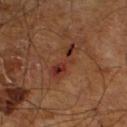notes: total-body-photography surveillance lesion; no biopsy
automated metrics: an area of roughly 6 mm² and an eccentricity of roughly 0.95; an average lesion color of about L≈31 a*≈24 b*≈27 (CIELAB) and a lesion-to-skin contrast of about 8.5 (normalized; higher = more distinct); a nevus-likeness score of about 0/100 and a lesion-detection confidence of about 100/100
lighting: cross-polarized illumination
site: the leg
lesion diameter: about 5 mm
imaging modality: ~15 mm crop, total-body skin-cancer survey
patient: male, in their mid- to late 60s